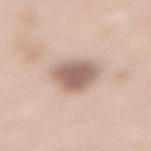| feature | finding |
|---|---|
| workup | imaged on a skin check; not biopsied |
| lesion diameter | about 4.5 mm |
| automated lesion analysis | a lesion area of about 12 mm², an outline eccentricity of about 0.6 (0 = round, 1 = elongated), and a symmetry-axis asymmetry near 0.2; an automated nevus-likeness rating near 60 out of 100 and a detector confidence of about 100 out of 100 that the crop contains a lesion |
| acquisition | total-body-photography crop, ~15 mm field of view |
| location | the lower back |
| illumination | white-light illumination |
| patient | male, aged 58–62 |